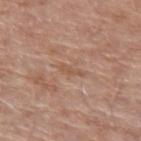Imaged during a routine full-body skin examination; the lesion was not biopsied and no histopathology is available. A close-up tile cropped from a whole-body skin photograph, about 15 mm across. The lesion is on the right upper arm. An algorithmic analysis of the crop reported a lesion area of about 2.5 mm², a shape eccentricity near 0.95, and two-axis asymmetry of about 0.35. This is a white-light tile. A male subject, aged around 70.